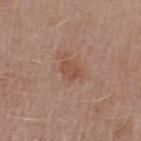image: total-body-photography crop, ~15 mm field of view; subject: male, about 70 years old; location: the left upper arm.